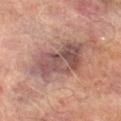follow-up: catalogued during a skin exam; not biopsied